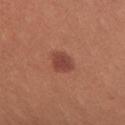Recorded during total-body skin imaging; not selected for excision or biopsy. A female subject approximately 35 years of age. From the upper back. Cropped from a total-body skin-imaging series; the visible field is about 15 mm. The tile uses white-light illumination.